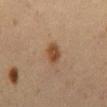notes — total-body-photography surveillance lesion; no biopsy